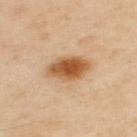An algorithmic analysis of the crop reported a footprint of about 11 mm², an eccentricity of roughly 0.75, and a shape-asymmetry score of about 0.1 (0 = symmetric). The software also gave a border-irregularity rating of about 1.5/10 and peripheral color asymmetry of about 1.5. It also reported a classifier nevus-likeness of about 100/100 and a lesion-detection confidence of about 100/100. This is a cross-polarized tile. A male patient, in their mid- to late 50s. Approximately 5 mm at its widest. On the back. A close-up tile cropped from a whole-body skin photograph, about 15 mm across.